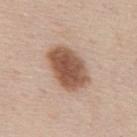Captured during whole-body skin photography for melanoma surveillance; the lesion was not biopsied. The subject is a male aged around 45. A roughly 15 mm field-of-view crop from a total-body skin photograph. The lesion is on the mid back. Automated tile analysis of the lesion measured an area of roughly 17 mm². It also reported a lesion color around L≈53 a*≈20 b*≈29 in CIELAB. And it measured a within-lesion color-variation index near 4.5/10 and peripheral color asymmetry of about 1.5. The tile uses white-light illumination. Longest diameter approximately 5.5 mm.